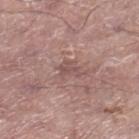notes: total-body-photography surveillance lesion; no biopsy
image source: ~15 mm crop, total-body skin-cancer survey
patient: male, aged 68–72
lighting: white-light illumination
site: the right lower leg
diameter: ~2.5 mm (longest diameter)
TBP lesion metrics: an area of roughly 4 mm², a shape eccentricity near 0.75, and a shape-asymmetry score of about 0.4 (0 = symmetric); a lesion–skin lightness drop of about 7; a border-irregularity index near 4/10; lesion-presence confidence of about 65/100